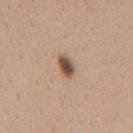Part of a total-body skin-imaging series; this lesion was reviewed on a skin check and was not flagged for biopsy.
The lesion is located on the mid back.
A 15 mm crop from a total-body photograph taken for skin-cancer surveillance.
About 3 mm across.
A male patient aged around 30.
Captured under white-light illumination.